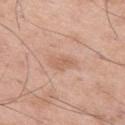Clinical summary: About 3.5 mm across. Automated image analysis of the tile measured a footprint of about 5.5 mm², an eccentricity of roughly 0.85, and a shape-asymmetry score of about 0.25 (0 = symmetric). It also reported roughly 7 lightness units darker than nearby skin and a normalized border contrast of about 5. A lesion tile, about 15 mm wide, cut from a 3D total-body photograph. The patient is a male approximately 50 years of age. On the left thigh. This is a white-light tile.